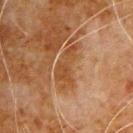Assessment:
Part of a total-body skin-imaging series; this lesion was reviewed on a skin check and was not flagged for biopsy.
Image and clinical context:
A male subject, approximately 80 years of age. The lesion is on the left upper arm. Approximately 5.5 mm at its widest. This is a cross-polarized tile. A lesion tile, about 15 mm wide, cut from a 3D total-body photograph.